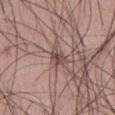The lesion was photographed on a routine skin check and not biopsied; there is no pathology result.
The subject is a male aged approximately 25.
An algorithmic analysis of the crop reported a footprint of about 3.5 mm², an outline eccentricity of about 0.8 (0 = round, 1 = elongated), and a symmetry-axis asymmetry near 0.3. And it measured border irregularity of about 3.5 on a 0–10 scale and radial color variation of about 1.
The tile uses white-light illumination.
About 2.5 mm across.
On the left thigh.
A lesion tile, about 15 mm wide, cut from a 3D total-body photograph.